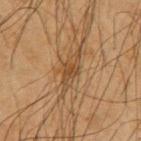No biopsy was performed on this lesion — it was imaged during a full skin examination and was not determined to be concerning. Located on the left upper arm. A male patient aged 58–62. This image is a 15 mm lesion crop taken from a total-body photograph. The lesion-visualizer software estimated a lesion area of about 6 mm² and two-axis asymmetry of about 0.25. It also reported about 8 CIELAB-L* units darker than the surrounding skin and a normalized border contrast of about 7. The software also gave a border-irregularity rating of about 3.5/10, a color-variation rating of about 3/10, and radial color variation of about 1. Approximately 4.5 mm at its widest.